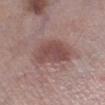  biopsy_status: not biopsied; imaged during a skin examination
  site: left lower leg
  patient:
    sex: male
    age_approx: 55
  image:
    source: total-body photography crop
    field_of_view_mm: 15
  lesion_size:
    long_diameter_mm_approx: 4.5